Imaged with cross-polarized lighting · a close-up tile cropped from a whole-body skin photograph, about 15 mm across · located on the left arm · a female patient, aged 48 to 52: 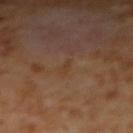Q: What did the biopsy show?
A: a lichen planus-like keratosis